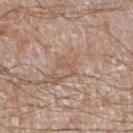Clinical summary:
This is a white-light tile. The lesion's longest dimension is about 2.5 mm. Automated image analysis of the tile measured a color-variation rating of about 0/10 and a peripheral color-asymmetry measure near 0. A 15 mm close-up tile from a total-body photography series done for melanoma screening. A male patient, in their 60s. The lesion is on the left lower leg.